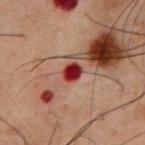Part of a total-body skin-imaging series; this lesion was reviewed on a skin check and was not flagged for biopsy. A male patient, aged 58–62. From the chest. A close-up tile cropped from a whole-body skin photograph, about 15 mm across. The recorded lesion diameter is about 2.5 mm. The tile uses cross-polarized illumination.